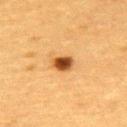Imaged during a routine full-body skin examination; the lesion was not biopsied and no histopathology is available.
This is a cross-polarized tile.
A female patient, aged around 55.
The lesion is on the upper back.
A 15 mm close-up extracted from a 3D total-body photography capture.
An algorithmic analysis of the crop reported a lesion area of about 5 mm² and an outline eccentricity of about 0.7 (0 = round, 1 = elongated). The analysis additionally found a mean CIELAB color near L≈42 a*≈22 b*≈38, a lesion–skin lightness drop of about 16, and a lesion-to-skin contrast of about 12 (normalized; higher = more distinct).